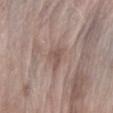| feature | finding |
|---|---|
| workup | total-body-photography surveillance lesion; no biopsy |
| lesion size | ~2.5 mm (longest diameter) |
| patient | female, about 75 years old |
| tile lighting | white-light illumination |
| image source | total-body-photography crop, ~15 mm field of view |
| site | the arm |